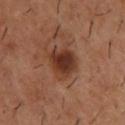{
  "biopsy_status": "not biopsied; imaged during a skin examination",
  "site": "upper back",
  "lesion_size": {
    "long_diameter_mm_approx": 4.0
  },
  "image": {
    "source": "total-body photography crop",
    "field_of_view_mm": 15
  },
  "patient": {
    "sex": "male",
    "age_approx": 55
  }
}